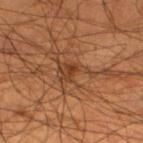A male subject, roughly 55 years of age.
The lesion's longest dimension is about 5 mm.
The lesion is located on the left lower leg.
Imaged with cross-polarized lighting.
A close-up tile cropped from a whole-body skin photograph, about 15 mm across.
Automated tile analysis of the lesion measured a lesion area of about 7 mm², an outline eccentricity of about 0.8 (0 = round, 1 = elongated), and two-axis asymmetry of about 0.6. It also reported an average lesion color of about L≈38 a*≈21 b*≈31 (CIELAB) and roughly 8 lightness units darker than nearby skin. It also reported a border-irregularity rating of about 8/10, a within-lesion color-variation index near 3.5/10, and peripheral color asymmetry of about 1. The analysis additionally found a classifier nevus-likeness of about 0/100 and a lesion-detection confidence of about 50/100.